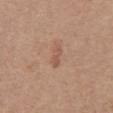Imaged during a routine full-body skin examination; the lesion was not biopsied and no histopathology is available.
Located on the abdomen.
A 15 mm close-up tile from a total-body photography series done for melanoma screening.
A female subject, roughly 65 years of age.
The tile uses white-light illumination.